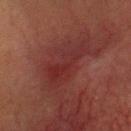Case summary:
- lesion diameter — ≈6.5 mm
- patient — male, roughly 70 years of age
- image source — ~15 mm tile from a whole-body skin photo
- location — the head or neck
- illumination — cross-polarized